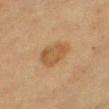{"image": {"source": "total-body photography crop", "field_of_view_mm": 15}, "site": "leg", "patient": {"sex": "female", "age_approx": 40}, "lighting": "cross-polarized", "lesion_size": {"long_diameter_mm_approx": 4.5}}